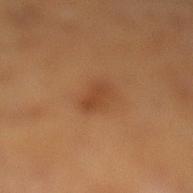notes — no biopsy performed (imaged during a skin exam) | automated lesion analysis — an area of roughly 4 mm² and two-axis asymmetry of about 0.3; about 7 CIELAB-L* units darker than the surrounding skin and a normalized lesion–skin contrast near 6; a border-irregularity rating of about 3/10 and radial color variation of about 0.5 | anatomic site — the left lower leg | size — about 3 mm | patient — male, aged 53–57 | image source — total-body-photography crop, ~15 mm field of view | illumination — cross-polarized illumination.